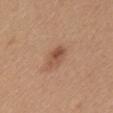{"biopsy_status": "not biopsied; imaged during a skin examination", "patient": {"sex": "female", "age_approx": 55}, "automated_metrics": {"vs_skin_darker_L": 10.0, "vs_skin_contrast_norm": 7.0, "color_variation_0_10": 3.5, "peripheral_color_asymmetry": 1.0, "nevus_likeness_0_100": 70, "lesion_detection_confidence_0_100": 100}, "image": {"source": "total-body photography crop", "field_of_view_mm": 15}, "lighting": "white-light", "site": "upper back"}